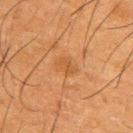The lesion was tiled from a total-body skin photograph and was not biopsied. The lesion's longest dimension is about 3 mm. Captured under cross-polarized illumination. A male patient, aged 33–37. A lesion tile, about 15 mm wide, cut from a 3D total-body photograph. An algorithmic analysis of the crop reported an area of roughly 4 mm², an eccentricity of roughly 0.75, and a shape-asymmetry score of about 0.3 (0 = symmetric). It also reported a mean CIELAB color near L≈42 a*≈20 b*≈34 and roughly 5 lightness units darker than nearby skin. The analysis additionally found a border-irregularity rating of about 3/10 and a peripheral color-asymmetry measure near 0.5. The software also gave a nevus-likeness score of about 5/100. From the upper back.